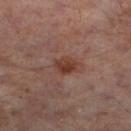Case summary:
• notes · total-body-photography surveillance lesion; no biopsy
• lighting · cross-polarized illumination
• location · the leg
• lesion size · ~2.5 mm (longest diameter)
• patient · male, aged 63 to 67
• imaging modality · ~15 mm crop, total-body skin-cancer survey